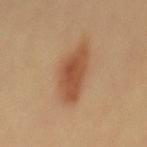notes: total-body-photography surveillance lesion; no biopsy | subject: female, aged around 50 | automated lesion analysis: an average lesion color of about L≈50 a*≈22 b*≈34 (CIELAB), about 11 CIELAB-L* units darker than the surrounding skin, and a normalized lesion–skin contrast near 8; border irregularity of about 3 on a 0–10 scale and radial color variation of about 1; a nevus-likeness score of about 100/100 and a detector confidence of about 100 out of 100 that the crop contains a lesion | acquisition: total-body-photography crop, ~15 mm field of view.